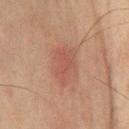The lesion was tiled from a total-body skin photograph and was not biopsied. Measured at roughly 4.5 mm in maximum diameter. A region of skin cropped from a whole-body photographic capture, roughly 15 mm wide. The patient is a male roughly 65 years of age. Automated tile analysis of the lesion measured an area of roughly 9 mm², a shape eccentricity near 0.8, and two-axis asymmetry of about 0.3. It also reported internal color variation of about 2 on a 0–10 scale and radial color variation of about 0.5. From the chest.